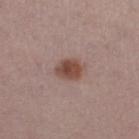Case summary:
* subject — male, roughly 65 years of age
* acquisition — ~15 mm crop, total-body skin-cancer survey
* lesion size — about 3 mm
* illumination — white-light illumination
* anatomic site — the right thigh
* automated metrics — an area of roughly 6.5 mm², an eccentricity of roughly 0.65, and a shape-asymmetry score of about 0.2 (0 = symmetric); a mean CIELAB color near L≈47 a*≈20 b*≈24, a lesion–skin lightness drop of about 12, and a normalized lesion–skin contrast near 9.5; peripheral color asymmetry of about 1